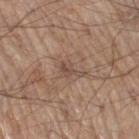| key | value |
|---|---|
| notes | total-body-photography surveillance lesion; no biopsy |
| acquisition | 15 mm crop, total-body photography |
| patient | male, roughly 70 years of age |
| location | the right thigh |
| image-analysis metrics | a shape eccentricity near 0.9 and a shape-asymmetry score of about 0.5 (0 = symmetric); an average lesion color of about L≈48 a*≈17 b*≈24 (CIELAB) and a normalized border contrast of about 6.5; a nevus-likeness score of about 0/100 and a detector confidence of about 100 out of 100 that the crop contains a lesion |
| lesion size | about 3 mm |